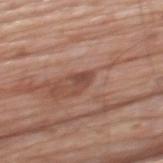No biopsy was performed on this lesion — it was imaged during a full skin examination and was not determined to be concerning. The recorded lesion diameter is about 2.5 mm. A male subject, aged 78 to 82. Located on the upper back. A roughly 15 mm field-of-view crop from a total-body skin photograph.